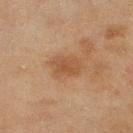biopsy status = catalogued during a skin exam; not biopsied | subject = female, in their 60s | imaging modality = 15 mm crop, total-body photography | anatomic site = the right lower leg | automated lesion analysis = a nevus-likeness score of about 5/100 and a detector confidence of about 100 out of 100 that the crop contains a lesion | lesion size = about 4 mm | lighting = cross-polarized.